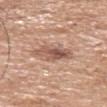Findings:
– notes: catalogued during a skin exam; not biopsied
– tile lighting: white-light illumination
– size: ≈4.5 mm
– subject: female, aged around 75
– image source: 15 mm crop, total-body photography
– body site: the head or neck